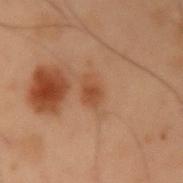Impression: The lesion was tiled from a total-body skin photograph and was not biopsied. Acquisition and patient details: The recorded lesion diameter is about 3 mm. On the arm. A 15 mm crop from a total-body photograph taken for skin-cancer surveillance. An algorithmic analysis of the crop reported an area of roughly 4.5 mm², an outline eccentricity of about 0.75 (0 = round, 1 = elongated), and a symmetry-axis asymmetry near 0.15. The analysis additionally found roughly 6 lightness units darker than nearby skin and a normalized lesion–skin contrast near 6. The software also gave a border-irregularity rating of about 1.5/10, a within-lesion color-variation index near 2.5/10, and peripheral color asymmetry of about 0.5. And it measured an automated nevus-likeness rating near 40 out of 100. A male patient, aged 53–57.